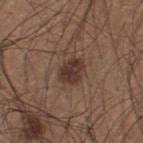Assessment:
Recorded during total-body skin imaging; not selected for excision or biopsy.
Image and clinical context:
The lesion is on the upper back. This image is a 15 mm lesion crop taken from a total-body photograph. Approximately 3 mm at its widest. This is a white-light tile. A male subject, aged 23 to 27.